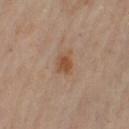follow-up: no biopsy performed (imaged during a skin exam) | lighting: cross-polarized | site: the left thigh | image source: total-body-photography crop, ~15 mm field of view | patient: female, in their 60s | lesion size: about 2.5 mm | TBP lesion metrics: a lesion area of about 4 mm² and a shape-asymmetry score of about 0.25 (0 = symmetric); a mean CIELAB color near L≈48 a*≈20 b*≈31 and a normalized lesion–skin contrast near 8; a border-irregularity rating of about 2.5/10 and a peripheral color-asymmetry measure near 1; an automated nevus-likeness rating near 85 out of 100 and lesion-presence confidence of about 100/100.